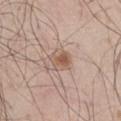{
  "biopsy_status": "not biopsied; imaged during a skin examination",
  "site": "leg",
  "patient": {
    "sex": "male",
    "age_approx": 45
  },
  "lighting": "white-light",
  "lesion_size": {
    "long_diameter_mm_approx": 3.0
  },
  "image": {
    "source": "total-body photography crop",
    "field_of_view_mm": 15
  }
}